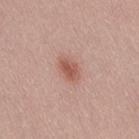Clinical summary:
The patient is a female in their mid- to late 20s. This is a white-light tile. The lesion is located on the right thigh. About 3 mm across. A lesion tile, about 15 mm wide, cut from a 3D total-body photograph.